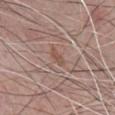Longest diameter approximately 2.5 mm. Imaged with white-light lighting. Located on the chest. The patient is a male aged around 85 or older. A 15 mm crop from a total-body photograph taken for skin-cancer surveillance.